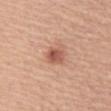<tbp_lesion>
  <biopsy_status>not biopsied; imaged during a skin examination</biopsy_status>
  <lesion_size>
    <long_diameter_mm_approx>2.5</long_diameter_mm_approx>
  </lesion_size>
  <patient>
    <sex>female</sex>
    <age_approx>55</age_approx>
  </patient>
  <image>
    <source>total-body photography crop</source>
    <field_of_view_mm>15</field_of_view_mm>
  </image>
  <site>abdomen</site>
  <automated_metrics>
    <area_mm2_approx>5.0</area_mm2_approx>
  </automated_metrics>
</tbp_lesion>